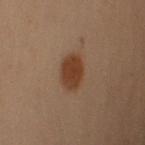The lesion was photographed on a routine skin check and not biopsied; there is no pathology result. The tile uses cross-polarized illumination. Automated image analysis of the tile measured an eccentricity of roughly 0.6. The software also gave a border-irregularity rating of about 1/10 and a color-variation rating of about 2/10. The analysis additionally found a nevus-likeness score of about 100/100 and a lesion-detection confidence of about 100/100. About 3.5 mm across. A male subject, roughly 55 years of age. A 15 mm close-up extracted from a 3D total-body photography capture. From the right upper arm.